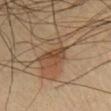notes = no biopsy performed (imaged during a skin exam) | lesion size = about 3 mm | automated metrics = an area of roughly 4.5 mm² and a symmetry-axis asymmetry near 0.45; a border-irregularity rating of about 4.5/10 and a peripheral color-asymmetry measure near 1; a nevus-likeness score of about 0/100 and a detector confidence of about 90 out of 100 that the crop contains a lesion | acquisition = ~15 mm crop, total-body skin-cancer survey | site = the chest | patient = male, aged around 50 | lighting = cross-polarized.